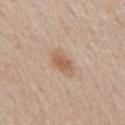Part of a total-body skin-imaging series; this lesion was reviewed on a skin check and was not flagged for biopsy. A 15 mm close-up extracted from a 3D total-body photography capture. Measured at roughly 4 mm in maximum diameter. A male patient aged around 60. From the mid back.